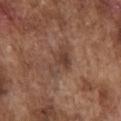Impression: Imaged during a routine full-body skin examination; the lesion was not biopsied and no histopathology is available. Image and clinical context: Captured under white-light illumination. The lesion's longest dimension is about 4.5 mm. Cropped from a total-body skin-imaging series; the visible field is about 15 mm. The lesion is located on the chest. The patient is a male aged 73–77.